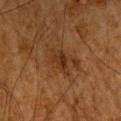Clinical impression: Recorded during total-body skin imaging; not selected for excision or biopsy. Context: Cropped from a total-body skin-imaging series; the visible field is about 15 mm. This is a cross-polarized tile. Automated tile analysis of the lesion measured a footprint of about 5 mm² and two-axis asymmetry of about 0.3. And it measured a mean CIELAB color near L≈26 a*≈18 b*≈28, roughly 7 lightness units darker than nearby skin, and a normalized lesion–skin contrast near 7.5. The recorded lesion diameter is about 3.5 mm. The patient is a male roughly 65 years of age. Located on the head or neck.